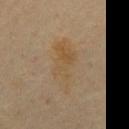* biopsy status: no biopsy performed (imaged during a skin exam)
* subject: male, roughly 60 years of age
* diameter: ~7.5 mm (longest diameter)
* acquisition: ~15 mm tile from a whole-body skin photo
* automated lesion analysis: a lesion–skin lightness drop of about 4; a nevus-likeness score of about 0/100
* body site: the mid back